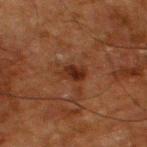{
  "biopsy_status": "not biopsied; imaged during a skin examination",
  "lighting": "cross-polarized",
  "patient": {
    "sex": "male",
    "age_approx": 80
  },
  "image": {
    "source": "total-body photography crop",
    "field_of_view_mm": 15
  },
  "site": "left thigh",
  "lesion_size": {
    "long_diameter_mm_approx": 3.0
  }
}